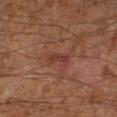Assessment:
No biopsy was performed on this lesion — it was imaged during a full skin examination and was not determined to be concerning.
Clinical summary:
Cropped from a whole-body photographic skin survey; the tile spans about 15 mm. The total-body-photography lesion software estimated a footprint of about 3 mm², an outline eccentricity of about 0.9 (0 = round, 1 = elongated), and a shape-asymmetry score of about 0.3 (0 = symmetric). And it measured roughly 6 lightness units darker than nearby skin and a normalized border contrast of about 6.5. And it measured a border-irregularity rating of about 3/10. Located on the right lower leg. This is a cross-polarized tile. A male subject, approximately 60 years of age. The lesion's longest dimension is about 2.5 mm.